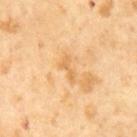| field | value |
|---|---|
| TBP lesion metrics | border irregularity of about 6 on a 0–10 scale, a color-variation rating of about 0/10, and radial color variation of about 0 |
| size | ~3 mm (longest diameter) |
| lighting | cross-polarized illumination |
| image source | ~15 mm tile from a whole-body skin photo |
| subject | male, aged 63–67 |